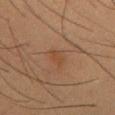Clinical impression:
This lesion was catalogued during total-body skin photography and was not selected for biopsy.
Acquisition and patient details:
The lesion is on the mid back. The lesion's longest dimension is about 2.5 mm. A male subject, in their mid-50s. The tile uses cross-polarized illumination. This image is a 15 mm lesion crop taken from a total-body photograph.